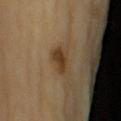<tbp_lesion>
<biopsy_status>not biopsied; imaged during a skin examination</biopsy_status>
<patient>
  <sex>female</sex>
  <age_approx>70</age_approx>
</patient>
<automated_metrics>
  <area_mm2_approx>5.5</area_mm2_approx>
  <shape_asymmetry>0.3</shape_asymmetry>
  <border_irregularity_0_10>2.5</border_irregularity_0_10>
</automated_metrics>
<site>arm</site>
<lighting>cross-polarized</lighting>
<image>
  <source>total-body photography crop</source>
  <field_of_view_mm>15</field_of_view_mm>
</image>
</tbp_lesion>